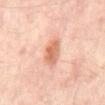Captured during whole-body skin photography for melanoma surveillance; the lesion was not biopsied.
A subject aged 53 to 57.
A close-up tile cropped from a whole-body skin photograph, about 15 mm across.
The total-body-photography lesion software estimated border irregularity of about 2.5 on a 0–10 scale and a color-variation rating of about 4.5/10.
Located on the mid back.
The tile uses cross-polarized illumination.